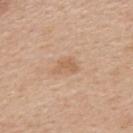Q: Is there a histopathology result?
A: imaged on a skin check; not biopsied
Q: Patient demographics?
A: male, aged 48–52
Q: Lesion size?
A: ≈3 mm
Q: What is the imaging modality?
A: total-body-photography crop, ~15 mm field of view
Q: Lesion location?
A: the upper back